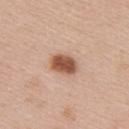Recorded during total-body skin imaging; not selected for excision or biopsy. This is a white-light tile. Cropped from a whole-body photographic skin survey; the tile spans about 15 mm. The lesion is on the right upper arm. The patient is a female approximately 35 years of age. The total-body-photography lesion software estimated a lesion area of about 7 mm², an outline eccentricity of about 0.7 (0 = round, 1 = elongated), and a symmetry-axis asymmetry near 0.1. Measured at roughly 3.5 mm in maximum diameter.